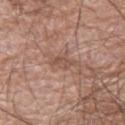lesion diameter = ~3 mm (longest diameter)
body site = the right lower leg
image source = ~15 mm tile from a whole-body skin photo
patient = male, aged 58 to 62